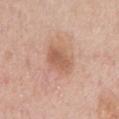Impression: Imaged during a routine full-body skin examination; the lesion was not biopsied and no histopathology is available. Image and clinical context: A male subject, aged 73 to 77. The tile uses white-light illumination. A lesion tile, about 15 mm wide, cut from a 3D total-body photograph. Longest diameter approximately 4 mm. From the chest.